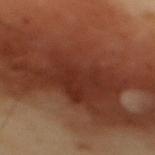<lesion>
<biopsy_status>not biopsied; imaged during a skin examination</biopsy_status>
<patient>
  <sex>male</sex>
  <age_approx>55</age_approx>
</patient>
<image>
  <source>total-body photography crop</source>
  <field_of_view_mm>15</field_of_view_mm>
</image>
<automated_metrics>
  <area_mm2_approx>115.0</area_mm2_approx>
  <eccentricity>0.9</eccentricity>
  <shape_asymmetry>0.2</shape_asymmetry>
  <border_irregularity_0_10>5.0</border_irregularity_0_10>
  <color_variation_0_10>5.5</color_variation_0_10>
  <peripheral_color_asymmetry>1.5</peripheral_color_asymmetry>
</automated_metrics>
<site>mid back</site>
</lesion>